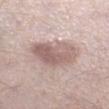Recorded during total-body skin imaging; not selected for excision or biopsy. About 5.5 mm across. A male patient, approximately 55 years of age. The tile uses white-light illumination. An algorithmic analysis of the crop reported a border-irregularity index near 2.5/10, a color-variation rating of about 5/10, and a peripheral color-asymmetry measure near 2. Cropped from a whole-body photographic skin survey; the tile spans about 15 mm. The lesion is located on the right lower leg.